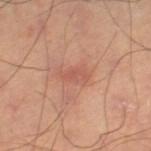Clinical impression:
The lesion was photographed on a routine skin check and not biopsied; there is no pathology result.
Background:
Measured at roughly 3 mm in maximum diameter. The lesion is located on the leg. The lesion-visualizer software estimated a border-irregularity rating of about 4/10. This is a cross-polarized tile. A roughly 15 mm field-of-view crop from a total-body skin photograph.